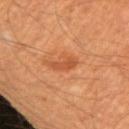biopsy status: no biopsy performed (imaged during a skin exam) | imaging modality: 15 mm crop, total-body photography | TBP lesion metrics: a mean CIELAB color near L≈48 a*≈27 b*≈38, about 8 CIELAB-L* units darker than the surrounding skin, and a lesion-to-skin contrast of about 6 (normalized; higher = more distinct); a border-irregularity index near 4.5/10, a within-lesion color-variation index near 0/10, and a peripheral color-asymmetry measure near 0 | patient: male, aged around 60 | anatomic site: the left upper arm | size: ≈3 mm.